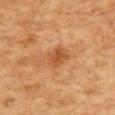Assessment: Captured during whole-body skin photography for melanoma surveillance; the lesion was not biopsied. Context: About 3.5 mm across. The lesion is on the upper back. The subject is a male about 80 years old. A 15 mm crop from a total-body photograph taken for skin-cancer surveillance.